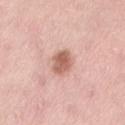Imaged during a routine full-body skin examination; the lesion was not biopsied and no histopathology is available. From the lower back. The subject is a female about 40 years old. The lesion's longest dimension is about 3.5 mm. This image is a 15 mm lesion crop taken from a total-body photograph. This is a white-light tile.